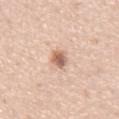Q: Was a biopsy performed?
A: imaged on a skin check; not biopsied
Q: Automated lesion metrics?
A: a classifier nevus-likeness of about 90/100 and lesion-presence confidence of about 100/100
Q: What are the patient's age and sex?
A: male, roughly 65 years of age
Q: What is the anatomic site?
A: the chest
Q: How was this image acquired?
A: 15 mm crop, total-body photography